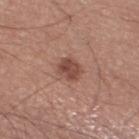Part of a total-body skin-imaging series; this lesion was reviewed on a skin check and was not flagged for biopsy. The lesion is on the leg. A 15 mm close-up extracted from a 3D total-body photography capture. The subject is a male in their 50s.